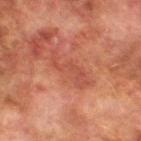follow-up — catalogued during a skin exam; not biopsied | TBP lesion metrics — a lesion area of about 6.5 mm² and an eccentricity of roughly 0.95; a classifier nevus-likeness of about 0/100 and a lesion-detection confidence of about 100/100 | acquisition — 15 mm crop, total-body photography | body site — the left lower leg | lighting — cross-polarized | lesion size — ~5.5 mm (longest diameter) | subject — male, roughly 75 years of age.